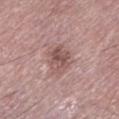Assessment: Imaged during a routine full-body skin examination; the lesion was not biopsied and no histopathology is available. Context: This image is a 15 mm lesion crop taken from a total-body photograph. The subject is a male aged around 75. The lesion is on the left lower leg. The total-body-photography lesion software estimated a lesion area of about 7.5 mm². It also reported a lesion color around L≈52 a*≈19 b*≈21 in CIELAB and a lesion–skin lightness drop of about 10. The software also gave a classifier nevus-likeness of about 5/100. This is a white-light tile.